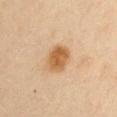Q: Was a biopsy performed?
A: no biopsy performed (imaged during a skin exam)
Q: Who is the patient?
A: male, approximately 35 years of age
Q: What is the lesion's diameter?
A: about 3.5 mm
Q: What lighting was used for the tile?
A: cross-polarized illumination
Q: Lesion location?
A: the right upper arm
Q: What is the imaging modality?
A: ~15 mm tile from a whole-body skin photo
Q: What did automated image analysis measure?
A: an average lesion color of about L≈52 a*≈19 b*≈36 (CIELAB), a lesion–skin lightness drop of about 11, and a lesion-to-skin contrast of about 9 (normalized; higher = more distinct); a classifier nevus-likeness of about 100/100 and a detector confidence of about 100 out of 100 that the crop contains a lesion